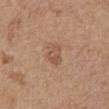workup: imaged on a skin check; not biopsied
lesion size: ≈3 mm
image source: total-body-photography crop, ~15 mm field of view
location: the front of the torso
patient: female, aged 63–67
tile lighting: white-light